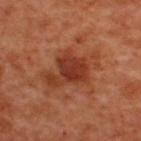This lesion was catalogued during total-body skin photography and was not selected for biopsy.